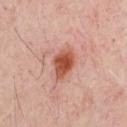No biopsy was performed on this lesion — it was imaged during a full skin examination and was not determined to be concerning. Approximately 3.5 mm at its widest. Captured under cross-polarized illumination. The lesion is on the chest. A patient in their mid- to late 50s. A lesion tile, about 15 mm wide, cut from a 3D total-body photograph.